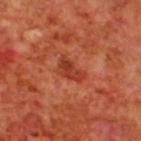Part of a total-body skin-imaging series; this lesion was reviewed on a skin check and was not flagged for biopsy. The lesion's longest dimension is about 3.5 mm. From the back. A male patient, aged around 70. A region of skin cropped from a whole-body photographic capture, roughly 15 mm wide.